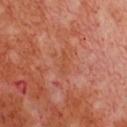Recorded during total-body skin imaging; not selected for excision or biopsy. A 15 mm crop from a total-body photograph taken for skin-cancer surveillance. The lesion is located on the chest. The tile uses cross-polarized illumination. An algorithmic analysis of the crop reported a lesion color around L≈51 a*≈30 b*≈36 in CIELAB, a lesion–skin lightness drop of about 5, and a normalized lesion–skin contrast near 5. It also reported border irregularity of about 3.5 on a 0–10 scale, a color-variation rating of about 0/10, and a peripheral color-asymmetry measure near 0. The analysis additionally found an automated nevus-likeness rating near 0 out of 100 and a detector confidence of about 95 out of 100 that the crop contains a lesion. The subject is a male aged around 55.